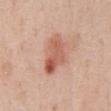Part of a total-body skin-imaging series; this lesion was reviewed on a skin check and was not flagged for biopsy.
A male patient in their mid-50s.
A close-up tile cropped from a whole-body skin photograph, about 15 mm across.
On the abdomen.